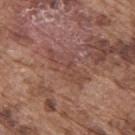Notes:
* workup — catalogued during a skin exam; not biopsied
* lighting — white-light
* patient — male, in their mid- to late 70s
* site — the upper back
* acquisition — total-body-photography crop, ~15 mm field of view
* TBP lesion metrics — an average lesion color of about L≈46 a*≈21 b*≈25 (CIELAB), roughly 6 lightness units darker than nearby skin, and a normalized lesion–skin contrast near 4.5